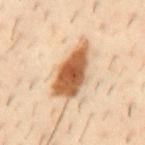The total-body-photography lesion software estimated border irregularity of about 3.5 on a 0–10 scale, a within-lesion color-variation index near 5.5/10, and a peripheral color-asymmetry measure near 1.5. It also reported a nevus-likeness score of about 100/100 and lesion-presence confidence of about 100/100.
The lesion is located on the mid back.
A male subject about 40 years old.
A 15 mm close-up tile from a total-body photography series done for melanoma screening.
This is a cross-polarized tile.
Approximately 7 mm at its widest.